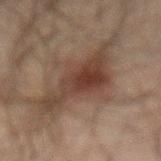Q: Was this lesion biopsied?
A: total-body-photography surveillance lesion; no biopsy
Q: What is the anatomic site?
A: the front of the torso
Q: Lesion size?
A: ~10.5 mm (longest diameter)
Q: Patient demographics?
A: male, aged 58–62
Q: Illumination type?
A: cross-polarized illumination
Q: What is the imaging modality?
A: total-body-photography crop, ~15 mm field of view
Q: Automated lesion metrics?
A: a footprint of about 21 mm² and two-axis asymmetry of about 0.4; a mean CIELAB color near L≈30 a*≈14 b*≈20, a lesion–skin lightness drop of about 8, and a normalized lesion–skin contrast near 8.5